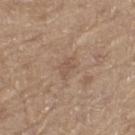Impression:
The lesion was tiled from a total-body skin photograph and was not biopsied.
Acquisition and patient details:
An algorithmic analysis of the crop reported a footprint of about 3 mm², an outline eccentricity of about 0.8 (0 = round, 1 = elongated), and a shape-asymmetry score of about 0.35 (0 = symmetric). The analysis additionally found a mean CIELAB color near L≈53 a*≈16 b*≈28 and about 6 CIELAB-L* units darker than the surrounding skin. The software also gave a border-irregularity rating of about 3.5/10, a within-lesion color-variation index near 1.5/10, and radial color variation of about 0.5. The lesion is on the left thigh. A lesion tile, about 15 mm wide, cut from a 3D total-body photograph. Measured at roughly 2.5 mm in maximum diameter. The subject is a male aged approximately 70. The tile uses white-light illumination.